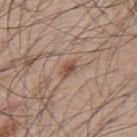  biopsy_status: not biopsied; imaged during a skin examination
  site: back
  image:
    source: total-body photography crop
    field_of_view_mm: 15
  automated_metrics:
    border_irregularity_0_10: 2.5
    color_variation_0_10: 3.0
    peripheral_color_asymmetry: 1.0
    nevus_likeness_0_100: 10
    lesion_detection_confidence_0_100: 100
  patient:
    sex: male
    age_approx: 55
  lighting: white-light
  lesion_size:
    long_diameter_mm_approx: 2.5